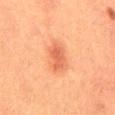workup=imaged on a skin check; not biopsied
patient=male, roughly 40 years of age
anatomic site=the mid back
acquisition=total-body-photography crop, ~15 mm field of view
size=~4.5 mm (longest diameter)